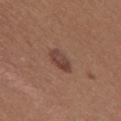Case summary:
* notes — imaged on a skin check; not biopsied
* image — ~15 mm tile from a whole-body skin photo
* site — the mid back
* lighting — white-light illumination
* patient — female, aged 23 to 27
* lesion size — ≈3.5 mm
* automated metrics — an average lesion color of about L≈42 a*≈20 b*≈25 (CIELAB) and a normalized border contrast of about 7.5; peripheral color asymmetry of about 1.5; an automated nevus-likeness rating near 75 out of 100